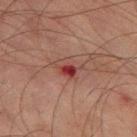notes: imaged on a skin check; not biopsied | illumination: cross-polarized illumination | diameter: about 3.5 mm | image source: 15 mm crop, total-body photography | body site: the left thigh | subject: male, in their mid-70s | automated metrics: a lesion area of about 5.5 mm², an eccentricity of roughly 0.8, and two-axis asymmetry of about 0.45; roughly 9 lightness units darker than nearby skin; internal color variation of about 10 on a 0–10 scale; a classifier nevus-likeness of about 0/100.